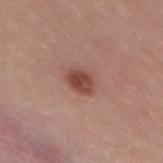Clinical impression: Part of a total-body skin-imaging series; this lesion was reviewed on a skin check and was not flagged for biopsy. Context: The lesion is located on the upper back. The subject is a female in their mid- to late 20s. Imaged with white-light lighting. A region of skin cropped from a whole-body photographic capture, roughly 15 mm wide. The total-body-photography lesion software estimated a border-irregularity rating of about 2/10. The analysis additionally found a classifier nevus-likeness of about 95/100 and lesion-presence confidence of about 100/100.